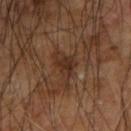Imaged with cross-polarized lighting.
A close-up tile cropped from a whole-body skin photograph, about 15 mm across.
An algorithmic analysis of the crop reported an area of roughly 7 mm², a shape eccentricity near 0.85, and a shape-asymmetry score of about 0.5 (0 = symmetric). The software also gave a color-variation rating of about 2/10. And it measured a nevus-likeness score of about 0/100 and lesion-presence confidence of about 90/100.
A male patient about 65 years old.
About 5 mm across.
Located on the right forearm.